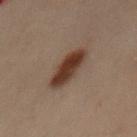follow-up = catalogued during a skin exam; not biopsied
site = the mid back
lighting = cross-polarized
diameter = ~5.5 mm (longest diameter)
patient = male, in their 50s
image = ~15 mm tile from a whole-body skin photo